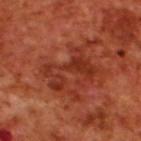A male patient, about 70 years old. A roughly 15 mm field-of-view crop from a total-body skin photograph. From the upper back.